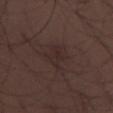Assessment: Imaged during a routine full-body skin examination; the lesion was not biopsied and no histopathology is available. Background: A male subject aged approximately 30. A region of skin cropped from a whole-body photographic capture, roughly 15 mm wide. The lesion is on the leg.